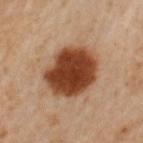<lesion>
<biopsy_status>not biopsied; imaged during a skin examination</biopsy_status>
<image>
  <source>total-body photography crop</source>
  <field_of_view_mm>15</field_of_view_mm>
</image>
<site>left upper arm</site>
<lesion_size>
  <long_diameter_mm_approx>6.0</long_diameter_mm_approx>
</lesion_size>
<patient>
  <sex>female</sex>
  <age_approx>55</age_approx>
</patient>
</lesion>